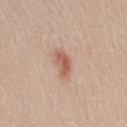Cropped from a total-body skin-imaging series; the visible field is about 15 mm. A female patient in their mid- to late 20s. Captured under white-light illumination. From the leg.